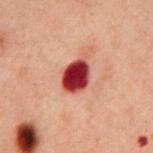No biopsy was performed on this lesion — it was imaged during a full skin examination and was not determined to be concerning.
Captured under cross-polarized illumination.
A male patient about 60 years old.
A roughly 15 mm field-of-view crop from a total-body skin photograph.
On the chest.